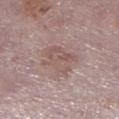Clinical impression:
Imaged during a routine full-body skin examination; the lesion was not biopsied and no histopathology is available.
Acquisition and patient details:
A lesion tile, about 15 mm wide, cut from a 3D total-body photograph. This is a white-light tile. The patient is a male aged around 75. On the right lower leg. The lesion-visualizer software estimated a lesion color around L≈54 a*≈17 b*≈20 in CIELAB, a lesion–skin lightness drop of about 7, and a lesion-to-skin contrast of about 5.5 (normalized; higher = more distinct). It also reported an automated nevus-likeness rating near 10 out of 100.